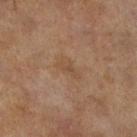Captured during whole-body skin photography for melanoma surveillance; the lesion was not biopsied.
A close-up tile cropped from a whole-body skin photograph, about 15 mm across.
The lesion's longest dimension is about 3 mm.
A female patient, in their 60s.
On the right lower leg.
The lesion-visualizer software estimated a footprint of about 3.5 mm², an eccentricity of roughly 0.9, and a shape-asymmetry score of about 0.35 (0 = symmetric). And it measured an average lesion color of about L≈41 a*≈15 b*≈28 (CIELAB), roughly 5 lightness units darker than nearby skin, and a normalized border contrast of about 5. It also reported a border-irregularity index near 4/10, internal color variation of about 0.5 on a 0–10 scale, and peripheral color asymmetry of about 0. It also reported a detector confidence of about 100 out of 100 that the crop contains a lesion.
This is a cross-polarized tile.